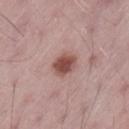Notes:
- follow-up — catalogued during a skin exam; not biopsied
- illumination — white-light illumination
- site — the left thigh
- patient — male, aged around 65
- lesion diameter — ≈3 mm
- image — ~15 mm crop, total-body skin-cancer survey